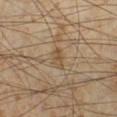A male patient, in their mid- to late 50s. Captured under cross-polarized illumination. Located on the left lower leg. The lesion-visualizer software estimated a border-irregularity index near 3.5/10, internal color variation of about 1 on a 0–10 scale, and radial color variation of about 0.5. And it measured a classifier nevus-likeness of about 0/100 and lesion-presence confidence of about 80/100. Cropped from a whole-body photographic skin survey; the tile spans about 15 mm. Longest diameter approximately 2.5 mm.